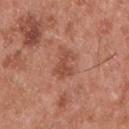Captured during whole-body skin photography for melanoma surveillance; the lesion was not biopsied.